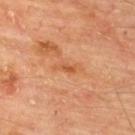biopsy_status: not biopsied; imaged during a skin examination
automated_metrics:
  area_mm2_approx: 2.5
  eccentricity: 0.9
  shape_asymmetry: 0.4
  cielab_L: 55
  cielab_a: 28
  cielab_b: 40
  vs_skin_contrast_norm: 6.0
  color_variation_0_10: 0.0
  peripheral_color_asymmetry: 0.0
  lesion_detection_confidence_0_100: 100
lighting: cross-polarized
site: upper back
image:
  source: total-body photography crop
  field_of_view_mm: 15
patient:
  sex: male
  age_approx: 65